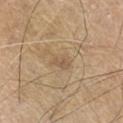Part of a total-body skin-imaging series; this lesion was reviewed on a skin check and was not flagged for biopsy.
Cropped from a total-body skin-imaging series; the visible field is about 15 mm.
An algorithmic analysis of the crop reported a lesion area of about 5 mm², a shape eccentricity near 0.75, and two-axis asymmetry of about 0.25. It also reported a mean CIELAB color near L≈56 a*≈14 b*≈32 and a lesion–skin lightness drop of about 6.
Located on the chest.
The recorded lesion diameter is about 3 mm.
This is a white-light tile.
The patient is a male aged 68 to 72.